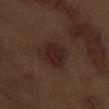Imaged during a routine full-body skin examination; the lesion was not biopsied and no histopathology is available.
A 15 mm close-up tile from a total-body photography series done for melanoma screening.
The patient is a male about 70 years old.
The lesion is on the left upper arm.